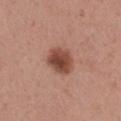patient:
  sex: female
  age_approx: 30
image:
  source: total-body photography crop
  field_of_view_mm: 15
lesion_size:
  long_diameter_mm_approx: 3.5
site: leg